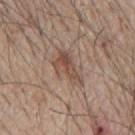Case summary:
- notes — imaged on a skin check; not biopsied
- location — the mid back
- patient — male, aged approximately 65
- diameter — ≈5 mm
- image source — ~15 mm crop, total-body skin-cancer survey